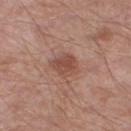Case summary:
• patient · male, aged approximately 55
• lesion diameter · ~3 mm (longest diameter)
• image source · total-body-photography crop, ~15 mm field of view
• illumination · white-light
• location · the left lower leg